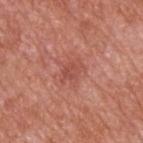- anatomic site · the upper back
- patient · male, aged approximately 65
- lighting · white-light
- image · ~15 mm crop, total-body skin-cancer survey
- diameter · ≈3 mm